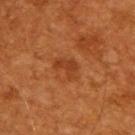Imaged during a routine full-body skin examination; the lesion was not biopsied and no histopathology is available.
From the back.
A male patient in their 60s.
A 15 mm close-up extracted from a 3D total-body photography capture.
Captured under cross-polarized illumination.
Automated image analysis of the tile measured a footprint of about 4.5 mm² and two-axis asymmetry of about 0.4. The analysis additionally found a border-irregularity rating of about 4.5/10, a color-variation rating of about 1/10, and a peripheral color-asymmetry measure near 0.5. It also reported an automated nevus-likeness rating near 0 out of 100.